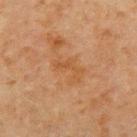biopsy status = imaged on a skin check; not biopsied | image source = ~15 mm tile from a whole-body skin photo | automated metrics = an average lesion color of about L≈43 a*≈20 b*≈34 (CIELAB), about 5 CIELAB-L* units darker than the surrounding skin, and a normalized border contrast of about 5; border irregularity of about 7.5 on a 0–10 scale, internal color variation of about 0 on a 0–10 scale, and peripheral color asymmetry of about 0; a classifier nevus-likeness of about 0/100 and a lesion-detection confidence of about 100/100 | subject = male, aged 68–72 | anatomic site = the right upper arm | size = ~3.5 mm (longest diameter).